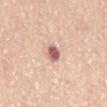No biopsy was performed on this lesion — it was imaged during a full skin examination and was not determined to be concerning.